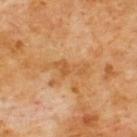Imaged during a routine full-body skin examination; the lesion was not biopsied and no histopathology is available.